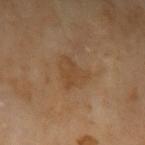Part of a total-body skin-imaging series; this lesion was reviewed on a skin check and was not flagged for biopsy. The lesion is on the arm. The subject is a male approximately 70 years of age. A lesion tile, about 15 mm wide, cut from a 3D total-body photograph.